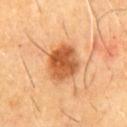Q: Is there a histopathology result?
A: imaged on a skin check; not biopsied